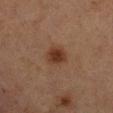Findings:
• tile lighting: cross-polarized illumination
• body site: the leg
• patient: male, aged 83 or older
• automated lesion analysis: a lesion–skin lightness drop of about 9 and a normalized lesion–skin contrast near 9; radial color variation of about 0.5
• image source: ~15 mm tile from a whole-body skin photo